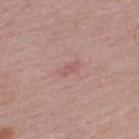<lesion>
  <biopsy_status>not biopsied; imaged during a skin examination</biopsy_status>
  <patient>
    <sex>male</sex>
    <age_approx>65</age_approx>
  </patient>
  <lighting>white-light</lighting>
  <image>
    <source>total-body photography crop</source>
    <field_of_view_mm>15</field_of_view_mm>
  </image>
  <automated_metrics>
    <area_mm2_approx>2.5</area_mm2_approx>
    <eccentricity>0.9</eccentricity>
    <shape_asymmetry>0.3</shape_asymmetry>
    <vs_skin_darker_L>6.0</vs_skin_darker_L>
    <vs_skin_contrast_norm>4.5</vs_skin_contrast_norm>
    <color_variation_0_10>0.0</color_variation_0_10>
    <peripheral_color_asymmetry>0.0</peripheral_color_asymmetry>
  </automated_metrics>
  <site>upper back</site>
</lesion>